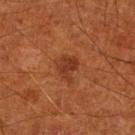Clinical impression:
The lesion was photographed on a routine skin check and not biopsied; there is no pathology result.
Context:
The lesion's longest dimension is about 3.5 mm. This is a cross-polarized tile. A close-up tile cropped from a whole-body skin photograph, about 15 mm across. The lesion is located on the right lower leg. A male subject aged approximately 65.